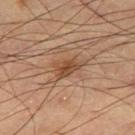Notes:
• biopsy status — total-body-photography surveillance lesion; no biopsy
• lesion size — ~3.5 mm (longest diameter)
• automated lesion analysis — a footprint of about 5.5 mm²; an average lesion color of about L≈47 a*≈20 b*≈32 (CIELAB), roughly 10 lightness units darker than nearby skin, and a normalized border contrast of about 8
• imaging modality — 15 mm crop, total-body photography
• illumination — cross-polarized illumination
• location — the leg
• patient — male, about 60 years old